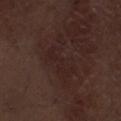Case summary:
• biopsy status: catalogued during a skin exam; not biopsied
• illumination: white-light illumination
• body site: the right thigh
• subject: male, aged 68–72
• diameter: about 6.5 mm
• acquisition: ~15 mm tile from a whole-body skin photo